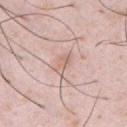workup: imaged on a skin check; not biopsied | lesion diameter: about 2.5 mm | patient: male, in their mid- to late 30s | anatomic site: the front of the torso | image source: ~15 mm tile from a whole-body skin photo.